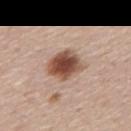{
  "biopsy_status": "not biopsied; imaged during a skin examination",
  "site": "mid back",
  "patient": {
    "sex": "male",
    "age_approx": 45
  },
  "image": {
    "source": "total-body photography crop",
    "field_of_view_mm": 15
  },
  "automated_metrics": {
    "eccentricity": 0.55,
    "shape_asymmetry": 0.2,
    "vs_skin_darker_L": 17.0,
    "vs_skin_contrast_norm": 12.0,
    "border_irregularity_0_10": 1.5,
    "color_variation_0_10": 6.5,
    "peripheral_color_asymmetry": 1.5,
    "nevus_likeness_0_100": 100,
    "lesion_detection_confidence_0_100": 100
  }
}